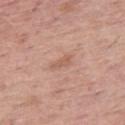biopsy status = total-body-photography surveillance lesion; no biopsy | image-analysis metrics = a border-irregularity rating of about 3/10, a within-lesion color-variation index near 1/10, and radial color variation of about 0.5; a detector confidence of about 100 out of 100 that the crop contains a lesion | patient = female, aged 58 to 62 | body site = the right thigh | image = 15 mm crop, total-body photography | illumination = white-light.